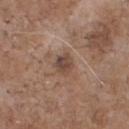Assessment:
Recorded during total-body skin imaging; not selected for excision or biopsy.
Clinical summary:
A male subject approximately 70 years of age. On the chest. The lesion-visualizer software estimated an area of roughly 4.5 mm², an outline eccentricity of about 0.45 (0 = round, 1 = elongated), and a shape-asymmetry score of about 0.25 (0 = symmetric). And it measured a mean CIELAB color near L≈45 a*≈17 b*≈25, roughly 10 lightness units darker than nearby skin, and a normalized lesion–skin contrast near 8. A 15 mm close-up tile from a total-body photography series done for melanoma screening. Imaged with white-light lighting.